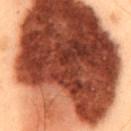The lesion was tiled from a total-body skin photograph and was not biopsied. A close-up tile cropped from a whole-body skin photograph, about 15 mm across. Imaged with cross-polarized lighting. The subject is a male aged 53 to 57. The lesion-visualizer software estimated a shape eccentricity near 0.8 and two-axis asymmetry of about 0.2. And it measured an average lesion color of about L≈38 a*≈24 b*≈29 (CIELAB), a lesion–skin lightness drop of about 28, and a normalized lesion–skin contrast near 19.5. The lesion is located on the mid back. About 20.5 mm across.